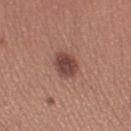Clinical impression: This lesion was catalogued during total-body skin photography and was not selected for biopsy. Clinical summary: A region of skin cropped from a whole-body photographic capture, roughly 15 mm wide. A female subject roughly 30 years of age. The lesion is on the right thigh. The lesion-visualizer software estimated an area of roughly 7 mm² and two-axis asymmetry of about 0.15. The software also gave an average lesion color of about L≈44 a*≈22 b*≈24 (CIELAB) and roughly 13 lightness units darker than nearby skin. The analysis additionally found border irregularity of about 1.5 on a 0–10 scale and internal color variation of about 3 on a 0–10 scale. The analysis additionally found an automated nevus-likeness rating near 85 out of 100 and a lesion-detection confidence of about 100/100. The tile uses white-light illumination.